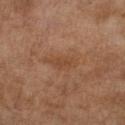Imaged during a routine full-body skin examination; the lesion was not biopsied and no histopathology is available. A 15 mm crop from a total-body photograph taken for skin-cancer surveillance. The total-body-photography lesion software estimated an outline eccentricity of about 0.9 (0 = round, 1 = elongated) and two-axis asymmetry of about 0.3. And it measured an average lesion color of about L≈39 a*≈18 b*≈28 (CIELAB), roughly 5 lightness units darker than nearby skin, and a normalized lesion–skin contrast near 4.5. Captured under cross-polarized illumination. A female subject, aged around 60. On the left lower leg. The lesion's longest dimension is about 4 mm.